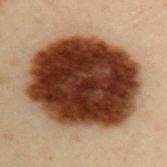Assessment:
Recorded during total-body skin imaging; not selected for excision or biopsy.
Background:
A male patient in their mid-50s. The lesion is located on the left upper arm. Cropped from a whole-body photographic skin survey; the tile spans about 15 mm. The lesion's longest dimension is about 11.5 mm.